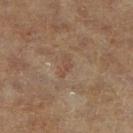Recorded during total-body skin imaging; not selected for excision or biopsy. Cropped from a whole-body photographic skin survey; the tile spans about 15 mm. A male patient approximately 85 years of age or older. Located on the right lower leg. Captured under cross-polarized illumination. The recorded lesion diameter is about 2.5 mm. Automated image analysis of the tile measured an average lesion color of about L≈45 a*≈17 b*≈27 (CIELAB), a lesion–skin lightness drop of about 5, and a normalized border contrast of about 4.5. The software also gave an automated nevus-likeness rating near 0 out of 100 and lesion-presence confidence of about 100/100.